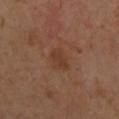Findings:
– biopsy status · catalogued during a skin exam; not biopsied